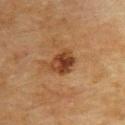A female patient aged 78–82.
Approximately 3.5 mm at its widest.
The tile uses cross-polarized illumination.
From the upper back.
A 15 mm close-up extracted from a 3D total-body photography capture.